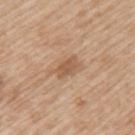follow-up: no biopsy performed (imaged during a skin exam) | location: the left upper arm | patient: male, aged approximately 65 | illumination: white-light | TBP lesion metrics: roughly 9 lightness units darker than nearby skin and a lesion-to-skin contrast of about 6.5 (normalized; higher = more distinct); a border-irregularity index near 2.5/10, a within-lesion color-variation index near 2/10, and radial color variation of about 0.5 | acquisition: 15 mm crop, total-body photography | lesion size: ~3 mm (longest diameter).